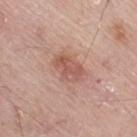Clinical impression:
The lesion was photographed on a routine skin check and not biopsied; there is no pathology result.
Clinical summary:
Captured under white-light illumination. The recorded lesion diameter is about 4 mm. An algorithmic analysis of the crop reported border irregularity of about 1.5 on a 0–10 scale and radial color variation of about 1. A male patient aged around 80. The lesion is located on the right thigh. A lesion tile, about 15 mm wide, cut from a 3D total-body photograph.